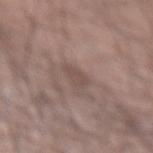workup — no biopsy performed (imaged during a skin exam) | patient — male, aged approximately 60 | automated lesion analysis — a shape-asymmetry score of about 0.25 (0 = symmetric); a lesion color around L≈48 a*≈16 b*≈20 in CIELAB and a normalized lesion–skin contrast near 5; a border-irregularity rating of about 2.5/10 and a color-variation rating of about 1/10 | size — about 2.5 mm | illumination — white-light illumination | location — the left forearm | imaging modality — ~15 mm tile from a whole-body skin photo.